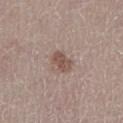This lesion was catalogued during total-body skin photography and was not selected for biopsy. A lesion tile, about 15 mm wide, cut from a 3D total-body photograph. The recorded lesion diameter is about 3 mm. The subject is a female roughly 50 years of age. The lesion is on the right lower leg.